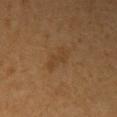follow-up: catalogued during a skin exam; not biopsied | lighting: cross-polarized | body site: the arm | automated lesion analysis: a lesion–skin lightness drop of about 5 and a normalized lesion–skin contrast near 5; border irregularity of about 4.5 on a 0–10 scale and radial color variation of about 0 | subject: female, in their 60s | lesion diameter: ~3.5 mm (longest diameter) | image: ~15 mm crop, total-body skin-cancer survey.